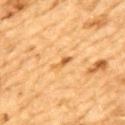workup: catalogued during a skin exam; not biopsied
size: about 3 mm
image: 15 mm crop, total-body photography
anatomic site: the mid back
patient: male, roughly 85 years of age or older